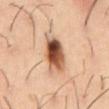acquisition — ~15 mm tile from a whole-body skin photo
patient — male, approximately 55 years of age
image-analysis metrics — a footprint of about 12 mm² and a shape-asymmetry score of about 0.2 (0 = symmetric); a lesion color around L≈52 a*≈22 b*≈33 in CIELAB, roughly 20 lightness units darker than nearby skin, and a normalized border contrast of about 12.5; border irregularity of about 2.5 on a 0–10 scale and peripheral color asymmetry of about 5.5; a nevus-likeness score of about 100/100
site — the abdomen